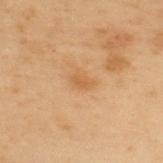No biopsy was performed on this lesion — it was imaged during a full skin examination and was not determined to be concerning.
From the upper back.
The recorded lesion diameter is about 2.5 mm.
A male subject aged 53–57.
A 15 mm close-up tile from a total-body photography series done for melanoma screening.